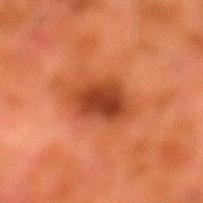body site: the left lower leg | TBP lesion metrics: a lesion area of about 12 mm², an eccentricity of roughly 0.75, and a shape-asymmetry score of about 0.25 (0 = symmetric); an automated nevus-likeness rating near 85 out of 100 | imaging modality: total-body-photography crop, ~15 mm field of view | subject: male, roughly 80 years of age | size: about 5 mm.